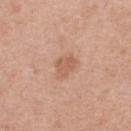Imaged during a routine full-body skin examination; the lesion was not biopsied and no histopathology is available.
A female subject, aged 38 to 42.
A 15 mm crop from a total-body photograph taken for skin-cancer surveillance.
Located on the upper back.
Captured under white-light illumination.